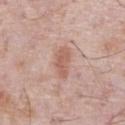Captured during whole-body skin photography for melanoma surveillance; the lesion was not biopsied. The recorded lesion diameter is about 4 mm. The subject is a male in their 70s. The total-body-photography lesion software estimated an area of roughly 6.5 mm², a shape eccentricity near 0.9, and a shape-asymmetry score of about 0.2 (0 = symmetric). It also reported a mean CIELAB color near L≈59 a*≈22 b*≈27, roughly 9 lightness units darker than nearby skin, and a lesion-to-skin contrast of about 6.5 (normalized; higher = more distinct). And it measured border irregularity of about 2.5 on a 0–10 scale, a within-lesion color-variation index near 2/10, and radial color variation of about 1. A 15 mm close-up tile from a total-body photography series done for melanoma screening. The lesion is located on the front of the torso. The tile uses white-light illumination.